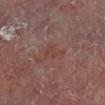<lesion>
  <biopsy_status>not biopsied; imaged during a skin examination</biopsy_status>
  <lesion_size>
    <long_diameter_mm_approx>3.0</long_diameter_mm_approx>
  </lesion_size>
  <site>right lower leg</site>
  <patient>
    <sex>male</sex>
    <age_approx>60</age_approx>
  </patient>
  <image>
    <source>total-body photography crop</source>
    <field_of_view_mm>15</field_of_view_mm>
  </image>
  <lighting>cross-polarized</lighting>
</lesion>